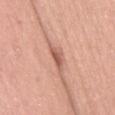<lesion>
  <biopsy_status>not biopsied; imaged during a skin examination</biopsy_status>
  <patient>
    <sex>female</sex>
    <age_approx>40</age_approx>
  </patient>
  <image>
    <source>total-body photography crop</source>
    <field_of_view_mm>15</field_of_view_mm>
  </image>
  <automated_metrics>
    <eccentricity>0.85</eccentricity>
    <cielab_L>57</cielab_L>
    <cielab_a>25</cielab_a>
    <cielab_b>29</cielab_b>
    <vs_skin_darker_L>12.0</vs_skin_darker_L>
    <lesion_detection_confidence_0_100>100</lesion_detection_confidence_0_100>
  </automated_metrics>
  <lighting>white-light</lighting>
  <lesion_size>
    <long_diameter_mm_approx>2.5</long_diameter_mm_approx>
  </lesion_size>
  <site>leg</site>
</lesion>